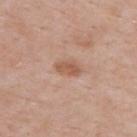| feature | finding |
|---|---|
| biopsy status | catalogued during a skin exam; not biopsied |
| illumination | white-light illumination |
| subject | male, aged 53–57 |
| imaging modality | ~15 mm crop, total-body skin-cancer survey |
| diameter | about 3 mm |
| anatomic site | the upper back |